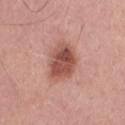Clinical impression:
Part of a total-body skin-imaging series; this lesion was reviewed on a skin check and was not flagged for biopsy.
Image and clinical context:
A lesion tile, about 15 mm wide, cut from a 3D total-body photograph. The lesion-visualizer software estimated a footprint of about 14 mm², an eccentricity of roughly 0.7, and two-axis asymmetry of about 0.15. And it measured a lesion color around L≈52 a*≈25 b*≈26 in CIELAB, a lesion–skin lightness drop of about 14, and a normalized lesion–skin contrast near 9. It also reported a border-irregularity index near 2/10. And it measured an automated nevus-likeness rating near 90 out of 100 and lesion-presence confidence of about 100/100. The lesion is on the chest. Measured at roughly 5 mm in maximum diameter. Captured under white-light illumination. A male patient, aged approximately 60.